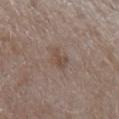Imaged during a routine full-body skin examination; the lesion was not biopsied and no histopathology is available. A female subject, in their mid-50s. A region of skin cropped from a whole-body photographic capture, roughly 15 mm wide. From the left forearm.